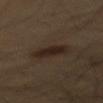Case summary:
– follow-up · total-body-photography surveillance lesion; no biopsy
– size · about 4.5 mm
– subject · male, about 55 years old
– body site · the mid back
– acquisition · 15 mm crop, total-body photography
– tile lighting · cross-polarized